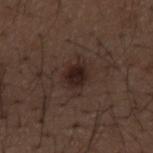The lesion was photographed on a routine skin check and not biopsied; there is no pathology result.
Approximately 3.5 mm at its widest.
A male patient approximately 50 years of age.
A roughly 15 mm field-of-view crop from a total-body skin photograph.
The lesion is on the mid back.